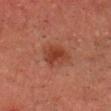| field | value |
|---|---|
| notes | catalogued during a skin exam; not biopsied |
| lighting | cross-polarized illumination |
| imaging modality | 15 mm crop, total-body photography |
| subject | male, about 45 years old |
| image-analysis metrics | an area of roughly 7 mm², an eccentricity of roughly 0.6, and a symmetry-axis asymmetry near 0.25; an average lesion color of about L≈33 a*≈24 b*≈27 (CIELAB), roughly 8 lightness units darker than nearby skin, and a normalized lesion–skin contrast near 7.5 |
| diameter | ≈3.5 mm |
| location | the head or neck |